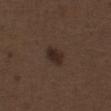Assessment:
Imaged during a routine full-body skin examination; the lesion was not biopsied and no histopathology is available.
Context:
Automated tile analysis of the lesion measured a footprint of about 4.5 mm², an outline eccentricity of about 0.7 (0 = round, 1 = elongated), and a shape-asymmetry score of about 0.25 (0 = symmetric). The software also gave a lesion color around L≈26 a*≈13 b*≈20 in CIELAB, roughly 8 lightness units darker than nearby skin, and a lesion-to-skin contrast of about 9 (normalized; higher = more distinct). The analysis additionally found a border-irregularity index near 2/10, a within-lesion color-variation index near 2/10, and a peripheral color-asymmetry measure near 0.5. The software also gave a classifier nevus-likeness of about 85/100 and a detector confidence of about 100 out of 100 that the crop contains a lesion. Measured at roughly 3 mm in maximum diameter. From the front of the torso. The patient is a male aged 68–72. A close-up tile cropped from a whole-body skin photograph, about 15 mm across.